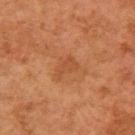Imaged during a routine full-body skin examination; the lesion was not biopsied and no histopathology is available.
Automated tile analysis of the lesion measured an area of roughly 4 mm², an eccentricity of roughly 0.8, and a shape-asymmetry score of about 0.4 (0 = symmetric). The analysis additionally found an average lesion color of about L≈44 a*≈24 b*≈35 (CIELAB), roughly 6 lightness units darker than nearby skin, and a normalized border contrast of about 4.5. The analysis additionally found an automated nevus-likeness rating near 0 out of 100 and a lesion-detection confidence of about 100/100.
The subject is a female aged approximately 60.
On the right upper arm.
The lesion's longest dimension is about 3 mm.
Cropped from a total-body skin-imaging series; the visible field is about 15 mm.
Imaged with cross-polarized lighting.